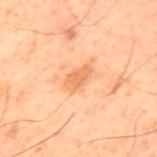biopsy_status: not biopsied; imaged during a skin examination
site: upper back
patient:
  sex: male
  age_approx: 60
image:
  source: total-body photography crop
  field_of_view_mm: 15
lighting: cross-polarized
lesion_size:
  long_diameter_mm_approx: 3.0
automated_metrics:
  border_irregularity_0_10: 2.0
  color_variation_0_10: 1.0
  peripheral_color_asymmetry: 0.5